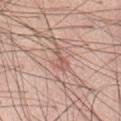Longest diameter approximately 3 mm.
Located on the abdomen.
Imaged with white-light lighting.
A male subject roughly 60 years of age.
A lesion tile, about 15 mm wide, cut from a 3D total-body photograph.